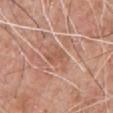The lesion was tiled from a total-body skin photograph and was not biopsied. Cropped from a whole-body photographic skin survey; the tile spans about 15 mm. On the front of the torso. The lesion-visualizer software estimated a mean CIELAB color near L≈55 a*≈22 b*≈31, roughly 7 lightness units darker than nearby skin, and a normalized lesion–skin contrast near 5.5. It also reported a within-lesion color-variation index near 1.5/10 and radial color variation of about 0.5. The patient is a male aged 58 to 62. The recorded lesion diameter is about 3 mm. Captured under white-light illumination.